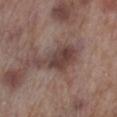Impression:
Part of a total-body skin-imaging series; this lesion was reviewed on a skin check and was not flagged for biopsy.
Image and clinical context:
A region of skin cropped from a whole-body photographic capture, roughly 15 mm wide. A male patient, aged around 70. Automated image analysis of the tile measured a footprint of about 12 mm² and two-axis asymmetry of about 0.4. The analysis additionally found a lesion color around L≈41 a*≈17 b*≈19 in CIELAB and roughly 10 lightness units darker than nearby skin. It also reported border irregularity of about 4.5 on a 0–10 scale and radial color variation of about 1. It also reported an automated nevus-likeness rating near 0 out of 100. The lesion is on the right lower leg. The recorded lesion diameter is about 6 mm.